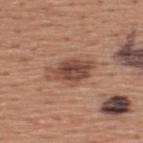| feature | finding |
|---|---|
| workup | no biopsy performed (imaged during a skin exam) |